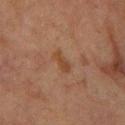The lesion was tiled from a total-body skin photograph and was not biopsied.